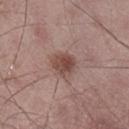Case summary:
* workup: catalogued during a skin exam; not biopsied
* site: the right lower leg
* patient: male, aged 63–67
* acquisition: 15 mm crop, total-body photography